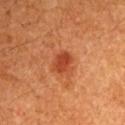Assessment: No biopsy was performed on this lesion — it was imaged during a full skin examination and was not determined to be concerning. Acquisition and patient details: The lesion is located on the left upper arm. A close-up tile cropped from a whole-body skin photograph, about 15 mm across. The lesion's longest dimension is about 3 mm. A male patient aged 58 to 62. This is a cross-polarized tile.